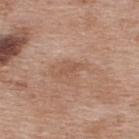Clinical impression: Part of a total-body skin-imaging series; this lesion was reviewed on a skin check and was not flagged for biopsy. Image and clinical context: A roughly 15 mm field-of-view crop from a total-body skin photograph. The subject is a female in their 40s. Captured under white-light illumination. Automated tile analysis of the lesion measured a lesion area of about 2.5 mm² and two-axis asymmetry of about 0.35. Measured at roughly 3 mm in maximum diameter. The lesion is located on the upper back.